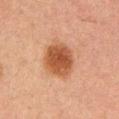The lesion was tiled from a total-body skin photograph and was not biopsied.
The lesion is on the arm.
A male patient, in their 50s.
Cropped from a total-body skin-imaging series; the visible field is about 15 mm.